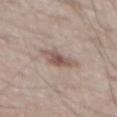This lesion was catalogued during total-body skin photography and was not selected for biopsy.
On the mid back.
The tile uses white-light illumination.
A male subject, approximately 55 years of age.
A 15 mm close-up tile from a total-body photography series done for melanoma screening.
About 4 mm across.